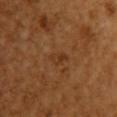notes = no biopsy performed (imaged during a skin exam); size = ~2.5 mm (longest diameter); subject = female, in their mid-50s; image source = ~15 mm crop, total-body skin-cancer survey; site = the arm.